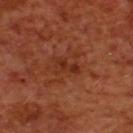| field | value |
|---|---|
| workup | total-body-photography surveillance lesion; no biopsy |
| tile lighting | cross-polarized illumination |
| imaging modality | 15 mm crop, total-body photography |
| anatomic site | the upper back |
| subject | male, in their 70s |